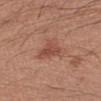No biopsy was performed on this lesion — it was imaged during a full skin examination and was not determined to be concerning.
A male subject, about 30 years old.
The lesion is located on the left upper arm.
Cropped from a whole-body photographic skin survey; the tile spans about 15 mm.
Approximately 4.5 mm at its widest.
Automated tile analysis of the lesion measured an area of roughly 9 mm² and an eccentricity of roughly 0.6. The software also gave a nevus-likeness score of about 55/100 and a detector confidence of about 100 out of 100 that the crop contains a lesion.
Imaged with white-light lighting.